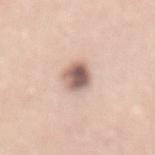  biopsy_status: not biopsied; imaged during a skin examination
  site: lower back
  image:
    source: total-body photography crop
    field_of_view_mm: 15
  patient:
    sex: male
    age_approx: 55
  automated_metrics:
    area_mm2_approx: 6.5
    eccentricity: 0.7